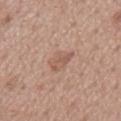Clinical impression:
The lesion was photographed on a routine skin check and not biopsied; there is no pathology result.
Clinical summary:
About 3.5 mm across. This is a white-light tile. The lesion is located on the mid back. A male patient aged around 65. A 15 mm close-up extracted from a 3D total-body photography capture.